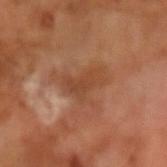lesion size = ~4 mm (longest diameter)
automated metrics = an average lesion color of about L≈43 a*≈23 b*≈32 (CIELAB), roughly 7 lightness units darker than nearby skin, and a normalized border contrast of about 6; an automated nevus-likeness rating near 0 out of 100 and a lesion-detection confidence of about 100/100
illumination = cross-polarized
patient = male, aged approximately 65
image source = ~15 mm crop, total-body skin-cancer survey